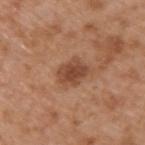follow-up: total-body-photography surveillance lesion; no biopsy
imaging modality: 15 mm crop, total-body photography
subject: male, aged around 65
location: the upper back
lesion diameter: about 3.5 mm
image-analysis metrics: a mean CIELAB color near L≈46 a*≈23 b*≈32 and a lesion-to-skin contrast of about 8 (normalized; higher = more distinct)